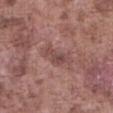About 4 mm across. This image is a 15 mm lesion crop taken from a total-body photograph. Automated image analysis of the tile measured an area of roughly 6 mm² and an outline eccentricity of about 0.85 (0 = round, 1 = elongated). And it measured a mean CIELAB color near L≈47 a*≈21 b*≈21, roughly 8 lightness units darker than nearby skin, and a normalized lesion–skin contrast near 6. And it measured a nevus-likeness score of about 0/100 and lesion-presence confidence of about 100/100. The patient is a male aged around 75. On the abdomen.